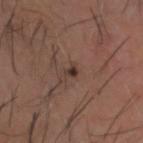Assessment:
This lesion was catalogued during total-body skin photography and was not selected for biopsy.
Image and clinical context:
This image is a 15 mm lesion crop taken from a total-body photograph. The lesion is on the head or neck. This is a cross-polarized tile. A male patient, in their mid-60s.